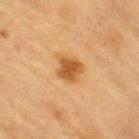A male patient, in their mid-80s or older.
A roughly 15 mm field-of-view crop from a total-body skin photograph.
Located on the right upper arm.
An algorithmic analysis of the crop reported an area of roughly 7.5 mm², an eccentricity of roughly 0.7, and two-axis asymmetry of about 0.25. It also reported an average lesion color of about L≈49 a*≈21 b*≈40 (CIELAB), a lesion–skin lightness drop of about 11, and a normalized border contrast of about 8.5. The software also gave an automated nevus-likeness rating near 95 out of 100 and lesion-presence confidence of about 100/100.